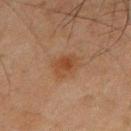Assessment: Part of a total-body skin-imaging series; this lesion was reviewed on a skin check and was not flagged for biopsy. Acquisition and patient details: A male patient, approximately 45 years of age. An algorithmic analysis of the crop reported an area of roughly 6.5 mm², an outline eccentricity of about 0.65 (0 = round, 1 = elongated), and a symmetry-axis asymmetry near 0.25. The software also gave a border-irregularity rating of about 2/10, internal color variation of about 3.5 on a 0–10 scale, and a peripheral color-asymmetry measure near 1. The analysis additionally found a nevus-likeness score of about 75/100. The lesion's longest dimension is about 3 mm. A roughly 15 mm field-of-view crop from a total-body skin photograph. The tile uses cross-polarized illumination. The lesion is located on the mid back.